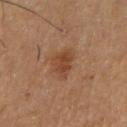Clinical impression: This lesion was catalogued during total-body skin photography and was not selected for biopsy. Acquisition and patient details: From the arm. This is a cross-polarized tile. A close-up tile cropped from a whole-body skin photograph, about 15 mm across. About 3.5 mm across. A male subject approximately 55 years of age.